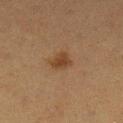patient: female, aged around 40
lesion diameter: about 2.5 mm
acquisition: ~15 mm tile from a whole-body skin photo
anatomic site: the left lower leg